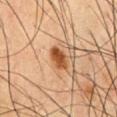Q: What is the imaging modality?
A: 15 mm crop, total-body photography
Q: Who is the patient?
A: male, aged 48 to 52
Q: What lighting was used for the tile?
A: cross-polarized
Q: Where on the body is the lesion?
A: the chest